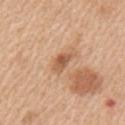Clinical impression:
Captured during whole-body skin photography for melanoma surveillance; the lesion was not biopsied.
Clinical summary:
This is a white-light tile. A male subject in their 60s. About 3 mm across. From the left upper arm. A region of skin cropped from a whole-body photographic capture, roughly 15 mm wide. The lesion-visualizer software estimated an outline eccentricity of about 0.8 (0 = round, 1 = elongated) and two-axis asymmetry of about 0.25. The analysis additionally found a lesion–skin lightness drop of about 11 and a normalized lesion–skin contrast near 7.5. It also reported a border-irregularity index near 2.5/10, internal color variation of about 3.5 on a 0–10 scale, and radial color variation of about 1.